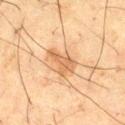The lesion was tiled from a total-body skin photograph and was not biopsied. Automated tile analysis of the lesion measured a footprint of about 7 mm² and a symmetry-axis asymmetry near 0.45. The software also gave a lesion color around L≈52 a*≈18 b*≈34 in CIELAB, a lesion–skin lightness drop of about 9, and a normalized lesion–skin contrast near 7. The software also gave a border-irregularity rating of about 4.5/10 and a peripheral color-asymmetry measure near 0.5. The analysis additionally found a classifier nevus-likeness of about 45/100 and a lesion-detection confidence of about 100/100. The subject is a male roughly 65 years of age. Captured under cross-polarized illumination. A roughly 15 mm field-of-view crop from a total-body skin photograph. The recorded lesion diameter is about 4 mm. On the right thigh.